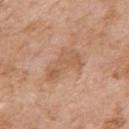Q: Was this lesion biopsied?
A: imaged on a skin check; not biopsied
Q: Who is the patient?
A: female, aged around 75
Q: What is the imaging modality?
A: 15 mm crop, total-body photography
Q: What is the anatomic site?
A: the right upper arm